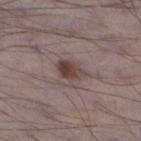Notes:
• biopsy status · catalogued during a skin exam; not biopsied
• site · the left lower leg
• automated lesion analysis · an outline eccentricity of about 0.7 (0 = round, 1 = elongated) and a shape-asymmetry score of about 0.25 (0 = symmetric); a border-irregularity rating of about 2.5/10
• acquisition · 15 mm crop, total-body photography
• subject · male, in their 70s
• lighting · white-light